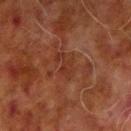{"biopsy_status": "not biopsied; imaged during a skin examination", "lesion_size": {"long_diameter_mm_approx": 3.5}, "automated_metrics": {"cielab_L": 27, "cielab_a": 21, "cielab_b": 26, "vs_skin_contrast_norm": 5.0, "color_variation_0_10": 1.5, "nevus_likeness_0_100": 0}, "lighting": "cross-polarized", "image": {"source": "total-body photography crop", "field_of_view_mm": 15}, "site": "right upper arm", "patient": {"sex": "male", "age_approx": 70}}